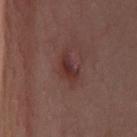Clinical summary: From the chest. A region of skin cropped from a whole-body photographic capture, roughly 15 mm wide. A female subject about 55 years old.